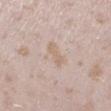| field | value |
|---|---|
| workup | no biopsy performed (imaged during a skin exam) |
| acquisition | ~15 mm tile from a whole-body skin photo |
| lesion diameter | ≈2.5 mm |
| anatomic site | the left lower leg |
| patient | female, approximately 25 years of age |